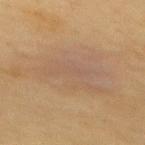No biopsy was performed on this lesion — it was imaged during a full skin examination and was not determined to be concerning. Imaged with cross-polarized lighting. Approximately 10.5 mm at its widest. A female subject aged approximately 40. The lesion-visualizer software estimated a lesion color around L≈49 a*≈13 b*≈27 in CIELAB, a lesion–skin lightness drop of about 4, and a normalized lesion–skin contrast near 4.5. The software also gave an automated nevus-likeness rating near 0 out of 100 and a lesion-detection confidence of about 75/100. A 15 mm crop from a total-body photograph taken for skin-cancer surveillance. On the mid back.